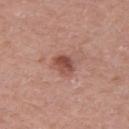{"biopsy_status": "not biopsied; imaged during a skin examination", "automated_metrics": {"shape_asymmetry": 0.2, "border_irregularity_0_10": 2.5, "color_variation_0_10": 5.0, "peripheral_color_asymmetry": 2.0}, "lesion_size": {"long_diameter_mm_approx": 3.0}, "image": {"source": "total-body photography crop", "field_of_view_mm": 15}, "site": "right upper arm", "patient": {"sex": "male", "age_approx": 50}}